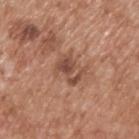  biopsy_status: not biopsied; imaged during a skin examination
  image:
    source: total-body photography crop
    field_of_view_mm: 15
  lighting: white-light
  patient:
    sex: male
    age_approx: 65
  site: upper back